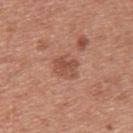biopsy_status: not biopsied; imaged during a skin examination
patient:
  sex: male
  age_approx: 30
image:
  source: total-body photography crop
  field_of_view_mm: 15
site: upper back